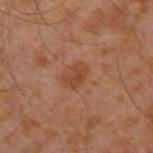Imaged during a routine full-body skin examination; the lesion was not biopsied and no histopathology is available.
The subject is a male in their 30s.
Located on the left upper arm.
The lesion's longest dimension is about 3 mm.
This image is a 15 mm lesion crop taken from a total-body photograph.
The total-body-photography lesion software estimated two-axis asymmetry of about 0.2. It also reported an average lesion color of about L≈43 a*≈23 b*≈33 (CIELAB) and about 7 CIELAB-L* units darker than the surrounding skin. The software also gave a border-irregularity rating of about 2.5/10, a within-lesion color-variation index near 2/10, and a peripheral color-asymmetry measure near 0.5. It also reported an automated nevus-likeness rating near 20 out of 100 and a lesion-detection confidence of about 100/100.